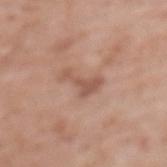Recorded during total-body skin imaging; not selected for excision or biopsy.
A female patient roughly 75 years of age.
A close-up tile cropped from a whole-body skin photograph, about 15 mm across.
Captured under white-light illumination.
The lesion is located on the mid back.
The lesion-visualizer software estimated an automated nevus-likeness rating near 0 out of 100.
The recorded lesion diameter is about 4 mm.